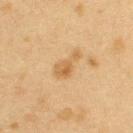biopsy status = imaged on a skin check; not biopsied
image = ~15 mm tile from a whole-body skin photo
patient = female, approximately 40 years of age
size = ≈4.5 mm
body site = the arm
tile lighting = cross-polarized illumination
image-analysis metrics = a mean CIELAB color near L≈51 a*≈15 b*≈36, roughly 7 lightness units darker than nearby skin, and a normalized lesion–skin contrast near 6.5; a border-irregularity index near 5/10, a color-variation rating of about 3/10, and peripheral color asymmetry of about 1; lesion-presence confidence of about 100/100